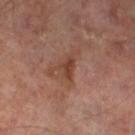Notes:
- workup · total-body-photography surveillance lesion; no biopsy
- lighting · cross-polarized
- site · the left thigh
- subject · male, approximately 65 years of age
- image · ~15 mm crop, total-body skin-cancer survey
- lesion diameter · ≈3 mm